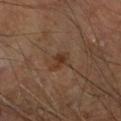Q: Was a biopsy performed?
A: total-body-photography surveillance lesion; no biopsy
Q: Illumination type?
A: cross-polarized illumination
Q: Who is the patient?
A: male, aged 68–72
Q: Where on the body is the lesion?
A: the right forearm
Q: Lesion size?
A: ≈2.5 mm
Q: What kind of image is this?
A: 15 mm crop, total-body photography